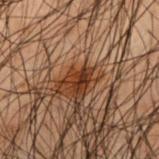No biopsy was performed on this lesion — it was imaged during a full skin examination and was not determined to be concerning. Imaged with cross-polarized lighting. The total-body-photography lesion software estimated a lesion color around L≈25 a*≈19 b*≈25 in CIELAB, roughly 10 lightness units darker than nearby skin, and a normalized lesion–skin contrast near 10.5. It also reported border irregularity of about 4.5 on a 0–10 scale, a within-lesion color-variation index near 3.5/10, and a peripheral color-asymmetry measure near 1. It also reported lesion-presence confidence of about 100/100. A region of skin cropped from a whole-body photographic capture, roughly 15 mm wide. Approximately 3 mm at its widest. The lesion is located on the mid back. The patient is a male roughly 50 years of age.